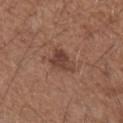Clinical impression: No biopsy was performed on this lesion — it was imaged during a full skin examination and was not determined to be concerning. Background: A male subject about 50 years old. A lesion tile, about 15 mm wide, cut from a 3D total-body photograph. Located on the left forearm. The tile uses white-light illumination.